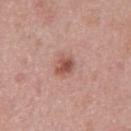Q: Is there a histopathology result?
A: total-body-photography surveillance lesion; no biopsy
Q: Patient demographics?
A: male, approximately 55 years of age
Q: What is the anatomic site?
A: the front of the torso
Q: What kind of image is this?
A: ~15 mm tile from a whole-body skin photo
Q: How was the tile lit?
A: white-light
Q: What is the lesion's diameter?
A: ≈2.5 mm